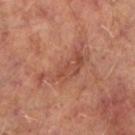Acquisition and patient details:
Longest diameter approximately 7 mm. The tile uses cross-polarized illumination. The lesion is on the leg. This image is a 15 mm lesion crop taken from a total-body photograph. The subject is a male aged 63 to 67. Automated image analysis of the tile measured a lesion color around L≈48 a*≈25 b*≈29 in CIELAB, roughly 8 lightness units darker than nearby skin, and a lesion-to-skin contrast of about 6 (normalized; higher = more distinct). The analysis additionally found a border-irregularity rating of about 8.5/10 and a within-lesion color-variation index near 3/10.